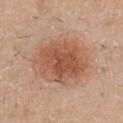Q: Was this lesion biopsied?
A: catalogued during a skin exam; not biopsied
Q: What is the lesion's diameter?
A: ~7 mm (longest diameter)
Q: How was this image acquired?
A: ~15 mm tile from a whole-body skin photo
Q: Patient demographics?
A: male, aged 38 to 42
Q: Where on the body is the lesion?
A: the chest